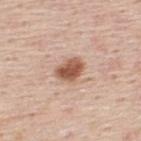Imaged during a routine full-body skin examination; the lesion was not biopsied and no histopathology is available.
The tile uses white-light illumination.
A 15 mm close-up extracted from a 3D total-body photography capture.
Automated tile analysis of the lesion measured a classifier nevus-likeness of about 100/100 and a detector confidence of about 100 out of 100 that the crop contains a lesion.
The patient is a male in their mid-40s.
On the upper back.
Measured at roughly 3.5 mm in maximum diameter.